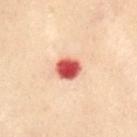biopsy_status: not biopsied; imaged during a skin examination
image:
  source: total-body photography crop
  field_of_view_mm: 15
patient:
  sex: female
  age_approx: 60
lighting: cross-polarized
site: abdomen